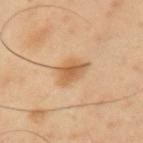Clinical impression:
The lesion was tiled from a total-body skin photograph and was not biopsied.
Background:
From the arm. A lesion tile, about 15 mm wide, cut from a 3D total-body photograph. Longest diameter approximately 3.5 mm. A male patient, roughly 40 years of age.